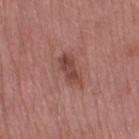Findings:
* biopsy status: imaged on a skin check; not biopsied
* body site: the right thigh
* image source: ~15 mm crop, total-body skin-cancer survey
* lighting: white-light illumination
* size: ~4 mm (longest diameter)
* patient: female, roughly 70 years of age
* TBP lesion metrics: a border-irregularity index near 3.5/10 and peripheral color asymmetry of about 1; a nevus-likeness score of about 10/100 and a lesion-detection confidence of about 100/100